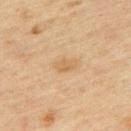Clinical impression:
No biopsy was performed on this lesion — it was imaged during a full skin examination and was not determined to be concerning.
Background:
A male patient, aged 43 to 47. Cropped from a whole-body photographic skin survey; the tile spans about 15 mm. On the back. This is a cross-polarized tile. Longest diameter approximately 2.5 mm.